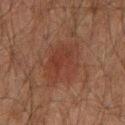Assessment: Part of a total-body skin-imaging series; this lesion was reviewed on a skin check and was not flagged for biopsy. Context: A male patient, approximately 60 years of age. Imaged with cross-polarized lighting. A region of skin cropped from a whole-body photographic capture, roughly 15 mm wide. Automated tile analysis of the lesion measured about 5 CIELAB-L* units darker than the surrounding skin. Located on the chest.